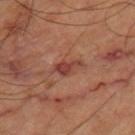follow-up — catalogued during a skin exam; not biopsied | patient — aged approximately 65 | location — the leg | image source — total-body-photography crop, ~15 mm field of view | automated lesion analysis — a lesion area of about 4 mm² and two-axis asymmetry of about 0.45; a classifier nevus-likeness of about 0/100 and a lesion-detection confidence of about 100/100.